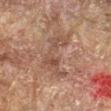Part of a total-body skin-imaging series; this lesion was reviewed on a skin check and was not flagged for biopsy. A male patient aged approximately 60. A 15 mm close-up extracted from a 3D total-body photography capture. The recorded lesion diameter is about 7 mm. The lesion-visualizer software estimated a lesion area of about 12 mm², a shape eccentricity near 0.95, and a symmetry-axis asymmetry near 0.65. And it measured a lesion color around L≈36 a*≈15 b*≈22 in CIELAB and a normalized lesion–skin contrast near 6. Located on the left forearm. Imaged with cross-polarized lighting.